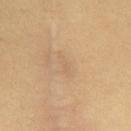  biopsy_status: not biopsied; imaged during a skin examination
  patient:
    sex: female
    age_approx: 25
  lighting: cross-polarized
  image:
    source: total-body photography crop
    field_of_view_mm: 15
  lesion_size:
    long_diameter_mm_approx: 3.0
  site: chest